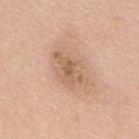notes — catalogued during a skin exam; not biopsied
anatomic site — the mid back
subject — male, aged 48 to 52
image-analysis metrics — an area of roughly 8.5 mm², an eccentricity of roughly 0.8, and a shape-asymmetry score of about 0.35 (0 = symmetric); a lesion color around L≈61 a*≈19 b*≈32 in CIELAB, a lesion–skin lightness drop of about 9, and a normalized border contrast of about 6
lesion size — ~5 mm (longest diameter)
acquisition — ~15 mm tile from a whole-body skin photo